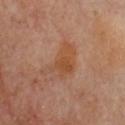The lesion was tiled from a total-body skin photograph and was not biopsied. On the chest. A female patient, aged approximately 60. An algorithmic analysis of the crop reported a footprint of about 10 mm² and an eccentricity of roughly 0.8. The software also gave a normalized lesion–skin contrast near 7. The software also gave border irregularity of about 7 on a 0–10 scale and internal color variation of about 2.5 on a 0–10 scale. Imaged with cross-polarized lighting. The lesion's longest dimension is about 5 mm. Cropped from a total-body skin-imaging series; the visible field is about 15 mm.